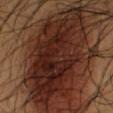This lesion was catalogued during total-body skin photography and was not selected for biopsy. An algorithmic analysis of the crop reported a lesion area of about 70 mm², an outline eccentricity of about 0.75 (0 = round, 1 = elongated), and a symmetry-axis asymmetry near 0.2. It also reported a classifier nevus-likeness of about 0/100 and a detector confidence of about 65 out of 100 that the crop contains a lesion. Measured at roughly 12.5 mm in maximum diameter. A 15 mm close-up extracted from a 3D total-body photography capture. The patient is a male roughly 55 years of age. The lesion is located on the arm.